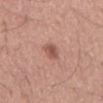Captured during whole-body skin photography for melanoma surveillance; the lesion was not biopsied.
A male patient aged approximately 55.
A roughly 15 mm field-of-view crop from a total-body skin photograph.
From the mid back.